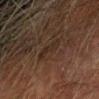The lesion was photographed on a routine skin check and not biopsied; there is no pathology result. The recorded lesion diameter is about 3 mm. Cropped from a whole-body photographic skin survey; the tile spans about 15 mm. On the left forearm. A male patient aged approximately 75. Captured under cross-polarized illumination.